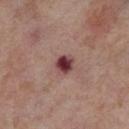Q: Was a biopsy performed?
A: imaged on a skin check; not biopsied
Q: Lesion size?
A: ≈2.5 mm
Q: What lighting was used for the tile?
A: cross-polarized
Q: Patient demographics?
A: female, in their mid-50s
Q: What is the imaging modality?
A: 15 mm crop, total-body photography
Q: Lesion location?
A: the right thigh